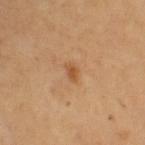biopsy status — imaged on a skin check; not biopsied
subject — female, aged approximately 65
lighting — cross-polarized
site — the right upper arm
image — ~15 mm tile from a whole-body skin photo